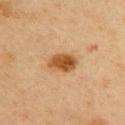Clinical impression: The lesion was tiled from a total-body skin photograph and was not biopsied. Image and clinical context: Captured under cross-polarized illumination. A 15 mm crop from a total-body photograph taken for skin-cancer surveillance. The patient is a female about 45 years old. The lesion is located on the upper back.